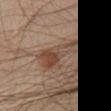The lesion was photographed on a routine skin check and not biopsied; there is no pathology result.
From the chest.
Imaged with white-light lighting.
A male subject aged around 60.
An algorithmic analysis of the crop reported a footprint of about 12 mm². And it measured a border-irregularity rating of about 5.5/10, internal color variation of about 7.5 on a 0–10 scale, and peripheral color asymmetry of about 2.5. It also reported lesion-presence confidence of about 100/100.
A lesion tile, about 15 mm wide, cut from a 3D total-body photograph.
The lesion's longest dimension is about 5.5 mm.